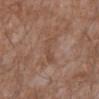biopsy status — catalogued during a skin exam; not biopsied | lesion size — ~4 mm (longest diameter) | TBP lesion metrics — an area of roughly 5 mm², a shape eccentricity near 0.95, and a shape-asymmetry score of about 0.45 (0 = symmetric); about 6 CIELAB-L* units darker than the surrounding skin and a lesion-to-skin contrast of about 4.5 (normalized; higher = more distinct); a border-irregularity index near 6/10 and peripheral color asymmetry of about 1.5 | anatomic site — the mid back | lighting — white-light illumination | image source — ~15 mm crop, total-body skin-cancer survey | patient — male, aged around 60.